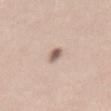The lesion was photographed on a routine skin check and not biopsied; there is no pathology result. Located on the mid back. A roughly 15 mm field-of-view crop from a total-body skin photograph. The tile uses white-light illumination. A female subject in their 40s. The lesion's longest dimension is about 3 mm.